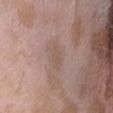biopsy_status: not biopsied; imaged during a skin examination
patient:
  sex: female
  age_approx: 25
lesion_size:
  long_diameter_mm_approx: 3.0
lighting: white-light
image:
  source: total-body photography crop
  field_of_view_mm: 15
site: head or neck